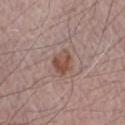Impression:
The lesion was photographed on a routine skin check and not biopsied; there is no pathology result.
Image and clinical context:
This is a white-light tile. About 3 mm across. A male subject aged around 70. A close-up tile cropped from a whole-body skin photograph, about 15 mm across. From the right forearm. Automated image analysis of the tile measured a shape eccentricity near 0.55. The analysis additionally found a lesion color around L≈49 a*≈19 b*≈25 in CIELAB and a lesion-to-skin contrast of about 8 (normalized; higher = more distinct). The software also gave a border-irregularity rating of about 2.5/10, a within-lesion color-variation index near 5/10, and a peripheral color-asymmetry measure near 1.5. It also reported an automated nevus-likeness rating near 85 out of 100 and a lesion-detection confidence of about 100/100.